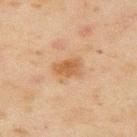Captured during whole-body skin photography for melanoma surveillance; the lesion was not biopsied. Cropped from a total-body skin-imaging series; the visible field is about 15 mm. The lesion's longest dimension is about 3 mm. A male subject, about 45 years old. Automated image analysis of the tile measured a border-irregularity rating of about 2.5/10 and a color-variation rating of about 3/10. Imaged with cross-polarized lighting. From the right upper arm.